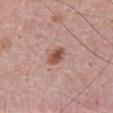– follow-up — no biopsy performed (imaged during a skin exam)
– lesion diameter — ≈2.5 mm
– image source — ~15 mm tile from a whole-body skin photo
– site — the abdomen
– subject — male, about 50 years old